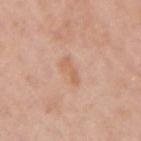Clinical impression:
This lesion was catalogued during total-body skin photography and was not selected for biopsy.
Image and clinical context:
The tile uses white-light illumination. A female subject in their mid-40s. From the right upper arm. Automated image analysis of the tile measured an eccentricity of roughly 0.9 and a shape-asymmetry score of about 0.45 (0 = symmetric). The software also gave a lesion–skin lightness drop of about 7. And it measured border irregularity of about 5 on a 0–10 scale and peripheral color asymmetry of about 0. The software also gave an automated nevus-likeness rating near 0 out of 100 and a lesion-detection confidence of about 100/100. The recorded lesion diameter is about 3 mm. A close-up tile cropped from a whole-body skin photograph, about 15 mm across.